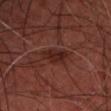notes = total-body-photography surveillance lesion; no biopsy | location = the front of the torso | patient = male, approximately 45 years of age | size = ~4.5 mm (longest diameter) | acquisition = ~15 mm crop, total-body skin-cancer survey | TBP lesion metrics = an average lesion color of about L≈23 a*≈22 b*≈22 (CIELAB), about 7 CIELAB-L* units darker than the surrounding skin, and a normalized lesion–skin contrast near 7.5; a color-variation rating of about 2.5/10.